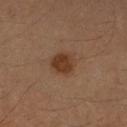Notes:
– biopsy status: no biopsy performed (imaged during a skin exam)
– diameter: about 3 mm
– image: ~15 mm crop, total-body skin-cancer survey
– automated lesion analysis: a footprint of about 7.5 mm², a shape eccentricity near 0.45, and two-axis asymmetry of about 0.15; roughly 9 lightness units darker than nearby skin; a border-irregularity rating of about 1/10, a within-lesion color-variation index near 2.5/10, and peripheral color asymmetry of about 1
– location: the right forearm
– patient: female, aged 53 to 57